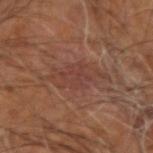Q: Was a biopsy performed?
A: imaged on a skin check; not biopsied